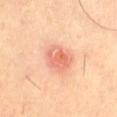Case summary:
- workup · no biopsy performed (imaged during a skin exam)
- tile lighting · cross-polarized illumination
- image-analysis metrics · about 11 CIELAB-L* units darker than the surrounding skin and a normalized border contrast of about 6.5; internal color variation of about 5 on a 0–10 scale and a peripheral color-asymmetry measure near 1.5; a nevus-likeness score of about 10/100 and a detector confidence of about 100 out of 100 that the crop contains a lesion
- subject · aged around 65
- lesion diameter · ~3.5 mm (longest diameter)
- site · the left thigh
- image · 15 mm crop, total-body photography